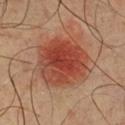biopsy_status: not biopsied; imaged during a skin examination
lesion_size:
  long_diameter_mm_approx: 6.5
image:
  source: total-body photography crop
  field_of_view_mm: 15
patient:
  sex: male
  age_approx: 40
site: front of the torso
automated_metrics:
  eccentricity: 0.3
  shape_asymmetry: 0.1
  border_irregularity_0_10: 1.5
  peripheral_color_asymmetry: 2.5